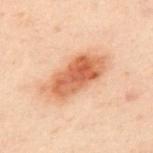Clinical impression: No biopsy was performed on this lesion — it was imaged during a full skin examination and was not determined to be concerning. Background: Cropped from a total-body skin-imaging series; the visible field is about 15 mm. The patient is a male about 50 years old. The recorded lesion diameter is about 7.5 mm. The lesion is located on the back.